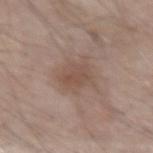image source — ~15 mm crop, total-body skin-cancer survey
lighting — white-light
patient — male, in their 70s
size — ~3.5 mm (longest diameter)
body site — the left forearm